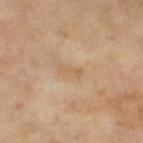No biopsy was performed on this lesion — it was imaged during a full skin examination and was not determined to be concerning. An algorithmic analysis of the crop reported a footprint of about 2.5 mm², an outline eccentricity of about 0.95 (0 = round, 1 = elongated), and two-axis asymmetry of about 0.35. And it measured a mean CIELAB color near L≈54 a*≈15 b*≈32, a lesion–skin lightness drop of about 6, and a normalized border contrast of about 5. The software also gave a border-irregularity index near 4.5/10 and a peripheral color-asymmetry measure near 0. The analysis additionally found a classifier nevus-likeness of about 0/100 and a lesion-detection confidence of about 100/100. A female patient, aged 58–62. The lesion is located on the left thigh. This is a cross-polarized tile. A 15 mm crop from a total-body photograph taken for skin-cancer surveillance. Longest diameter approximately 2.5 mm.